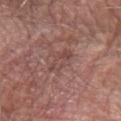An algorithmic analysis of the crop reported a classifier nevus-likeness of about 0/100.
A lesion tile, about 15 mm wide, cut from a 3D total-body photograph.
Captured under white-light illumination.
The lesion is on the left forearm.
About 3.5 mm across.
A male subject, about 80 years old.